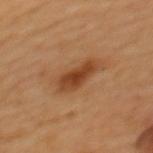Impression:
Imaged during a routine full-body skin examination; the lesion was not biopsied and no histopathology is available.
Acquisition and patient details:
A female patient in their mid- to late 40s. Located on the upper back. A lesion tile, about 15 mm wide, cut from a 3D total-body photograph.